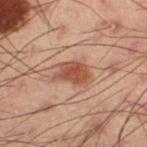notes: imaged on a skin check; not biopsied
acquisition: ~15 mm tile from a whole-body skin photo
site: the right thigh
size: about 3.5 mm
illumination: cross-polarized illumination
subject: male, in their mid-50s
TBP lesion metrics: a border-irregularity index near 3/10, a within-lesion color-variation index near 2.5/10, and radial color variation of about 1; a classifier nevus-likeness of about 90/100 and lesion-presence confidence of about 100/100